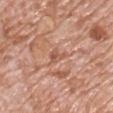Imaged during a routine full-body skin examination; the lesion was not biopsied and no histopathology is available. This is a white-light tile. A close-up tile cropped from a whole-body skin photograph, about 15 mm across. The lesion is on the chest. The subject is a male aged 68 to 72. The lesion's longest dimension is about 2.5 mm.